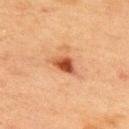Clinical impression:
Imaged during a routine full-body skin examination; the lesion was not biopsied and no histopathology is available.
Background:
From the upper back. Imaged with cross-polarized lighting. Cropped from a total-body skin-imaging series; the visible field is about 15 mm. A male subject roughly 70 years of age. An algorithmic analysis of the crop reported a shape-asymmetry score of about 0.3 (0 = symmetric). The analysis additionally found a mean CIELAB color near L≈43 a*≈26 b*≈34, about 14 CIELAB-L* units darker than the surrounding skin, and a lesion-to-skin contrast of about 10.5 (normalized; higher = more distinct). The analysis additionally found a nevus-likeness score of about 95/100 and a lesion-detection confidence of about 100/100. The lesion's longest dimension is about 3 mm.